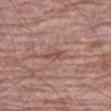Part of a total-body skin-imaging series; this lesion was reviewed on a skin check and was not flagged for biopsy.
The lesion is located on the leg.
Longest diameter approximately 3 mm.
A male patient aged 68 to 72.
Cropped from a whole-body photographic skin survey; the tile spans about 15 mm.